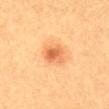<record>
  <biopsy_status>not biopsied; imaged during a skin examination</biopsy_status>
  <site>mid back</site>
  <image>
    <source>total-body photography crop</source>
    <field_of_view_mm>15</field_of_view_mm>
  </image>
  <lesion_size>
    <long_diameter_mm_approx>3.0</long_diameter_mm_approx>
  </lesion_size>
  <patient>
    <sex>female</sex>
    <age_approx>20</age_approx>
  </patient>
</record>